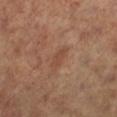No biopsy was performed on this lesion — it was imaged during a full skin examination and was not determined to be concerning. An algorithmic analysis of the crop reported an area of roughly 3.5 mm² and a shape eccentricity near 0.9. The analysis additionally found a lesion color around L≈45 a*≈21 b*≈29 in CIELAB and about 6 CIELAB-L* units darker than the surrounding skin. The analysis additionally found a border-irregularity rating of about 3/10, a within-lesion color-variation index near 1/10, and peripheral color asymmetry of about 0.5. A female subject, aged 63–67. The lesion is located on the leg. Captured under cross-polarized illumination. Approximately 3 mm at its widest. A lesion tile, about 15 mm wide, cut from a 3D total-body photograph.